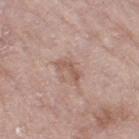No biopsy was performed on this lesion — it was imaged during a full skin examination and was not determined to be concerning. On the right thigh. The lesion's longest dimension is about 3 mm. Imaged with white-light lighting. A female patient aged approximately 70. Automated image analysis of the tile measured a shape eccentricity near 0.9 and a symmetry-axis asymmetry near 0.45. And it measured a color-variation rating of about 0/10 and peripheral color asymmetry of about 0. The analysis additionally found a nevus-likeness score of about 0/100 and a detector confidence of about 100 out of 100 that the crop contains a lesion. This image is a 15 mm lesion crop taken from a total-body photograph.